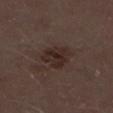Case summary:
– biopsy status — imaged on a skin check; not biopsied
– size — about 3.5 mm
– imaging modality — 15 mm crop, total-body photography
– TBP lesion metrics — a classifier nevus-likeness of about 15/100 and a lesion-detection confidence of about 100/100
– subject — male, about 70 years old
– illumination — white-light illumination
– body site — the left lower leg